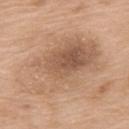  biopsy_status: not biopsied; imaged during a skin examination
  site: upper back
  lighting: white-light
  image:
    source: total-body photography crop
    field_of_view_mm: 15
  patient:
    sex: female
    age_approx: 75
  lesion_size:
    long_diameter_mm_approx: 11.0